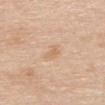Q: Is there a histopathology result?
A: total-body-photography surveillance lesion; no biopsy
Q: What is the imaging modality?
A: ~15 mm crop, total-body skin-cancer survey
Q: Lesion location?
A: the mid back
Q: Who is the patient?
A: female, approximately 65 years of age
Q: How large is the lesion?
A: ≈3 mm